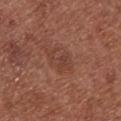Background: Automated image analysis of the tile measured an average lesion color of about L≈41 a*≈23 b*≈27 (CIELAB) and a lesion–skin lightness drop of about 6. Captured under white-light illumination. The lesion is on the front of the torso. This image is a 15 mm lesion crop taken from a total-body photograph. The patient is a female in their mid-70s.